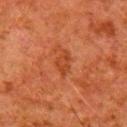biopsy status: imaged on a skin check; not biopsied | patient: male, aged 78 to 82 | image-analysis metrics: a border-irregularity index near 3.5/10, internal color variation of about 2 on a 0–10 scale, and a peripheral color-asymmetry measure near 0.5 | illumination: cross-polarized illumination | location: the right upper arm | diameter: about 3 mm | image: 15 mm crop, total-body photography.